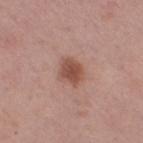The total-body-photography lesion software estimated an average lesion color of about L≈50 a*≈23 b*≈27 (CIELAB), a lesion–skin lightness drop of about 11, and a normalized lesion–skin contrast near 8.5. And it measured an automated nevus-likeness rating near 90 out of 100. A region of skin cropped from a whole-body photographic capture, roughly 15 mm wide. A female subject, in their mid-50s. About 3 mm across. From the right thigh.